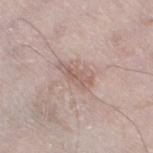On the left thigh. The total-body-photography lesion software estimated a lesion area of about 5.5 mm², an eccentricity of roughly 0.9, and two-axis asymmetry of about 0.3. And it measured border irregularity of about 4 on a 0–10 scale, internal color variation of about 3 on a 0–10 scale, and peripheral color asymmetry of about 1. The subject is a male roughly 65 years of age. The recorded lesion diameter is about 4 mm. A close-up tile cropped from a whole-body skin photograph, about 15 mm across. Captured under white-light illumination.